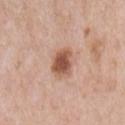Cropped from a total-body skin-imaging series; the visible field is about 15 mm. The tile uses white-light illumination. From the chest. The subject is a male aged around 60. The total-body-photography lesion software estimated a lesion color around L≈54 a*≈22 b*≈30 in CIELAB, a lesion–skin lightness drop of about 15, and a normalized lesion–skin contrast near 10. It also reported a border-irregularity rating of about 2.5/10 and a peripheral color-asymmetry measure near 1. The analysis additionally found a classifier nevus-likeness of about 100/100 and lesion-presence confidence of about 100/100.